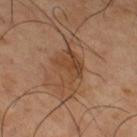Captured during whole-body skin photography for melanoma surveillance; the lesion was not biopsied. A close-up tile cropped from a whole-body skin photograph, about 15 mm across. About 6 mm across. From the right thigh. The patient is a male aged 63–67.